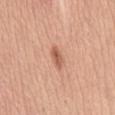{
  "biopsy_status": "not biopsied; imaged during a skin examination",
  "lesion_size": {
    "long_diameter_mm_approx": 3.0
  },
  "image": {
    "source": "total-body photography crop",
    "field_of_view_mm": 15
  },
  "lighting": "white-light",
  "site": "abdomen",
  "patient": {
    "sex": "female",
    "age_approx": 40
  }
}